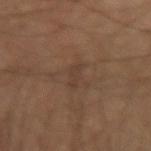Clinical impression:
The lesion was photographed on a routine skin check and not biopsied; there is no pathology result.
Context:
Longest diameter approximately 3 mm. The lesion is on the left forearm. Automated tile analysis of the lesion measured a lesion area of about 3 mm², an outline eccentricity of about 0.95 (0 = round, 1 = elongated), and two-axis asymmetry of about 0.4. The software also gave an average lesion color of about L≈35 a*≈14 b*≈24 (CIELAB) and a lesion-to-skin contrast of about 4.5 (normalized; higher = more distinct). And it measured a border-irregularity rating of about 4.5/10, a color-variation rating of about 0/10, and radial color variation of about 0. The analysis additionally found a classifier nevus-likeness of about 0/100 and a lesion-detection confidence of about 75/100. The tile uses cross-polarized illumination. A male patient, aged approximately 45. A 15 mm crop from a total-body photograph taken for skin-cancer surveillance.